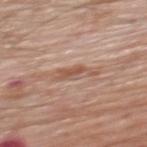biopsy status=catalogued during a skin exam; not biopsied
tile lighting=white-light
image source=total-body-photography crop, ~15 mm field of view
location=the upper back
patient=male, in their 80s